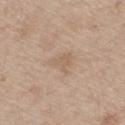The lesion was photographed on a routine skin check and not biopsied; there is no pathology result. This is a white-light tile. Cropped from a total-body skin-imaging series; the visible field is about 15 mm. A male subject aged around 70. The recorded lesion diameter is about 3.5 mm. The lesion is on the right thigh.